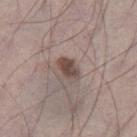Context: A male patient aged around 70. On the left thigh. A region of skin cropped from a whole-body photographic capture, roughly 15 mm wide.